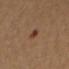automated_metrics:
  area_mm2_approx: 2.0
  eccentricity: 0.95
  cielab_L: 33
  cielab_a: 22
  cielab_b: 25
  vs_skin_darker_L: 10.0
  vs_skin_contrast_norm: 9.0
  color_variation_0_10: 0.0
  peripheral_color_asymmetry: 0.0
patient:
  sex: male
  age_approx: 65
lesion_size:
  long_diameter_mm_approx: 3.0
site: right upper arm
lighting: cross-polarized
image:
  source: total-body photography crop
  field_of_view_mm: 15